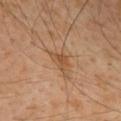No biopsy was performed on this lesion — it was imaged during a full skin examination and was not determined to be concerning. The subject is a male aged 48–52. Cropped from a whole-body photographic skin survey; the tile spans about 15 mm. The lesion is located on the back.